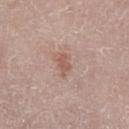<record>
  <biopsy_status>not biopsied; imaged during a skin examination</biopsy_status>
  <lesion_size>
    <long_diameter_mm_approx>2.5</long_diameter_mm_approx>
  </lesion_size>
  <image>
    <source>total-body photography crop</source>
    <field_of_view_mm>15</field_of_view_mm>
  </image>
  <automated_metrics>
    <shape_asymmetry>0.4</shape_asymmetry>
    <cielab_L>55</cielab_L>
    <cielab_a>20</cielab_a>
    <cielab_b>25</cielab_b>
    <vs_skin_darker_L>9.0</vs_skin_darker_L>
    <vs_skin_contrast_norm>6.0</vs_skin_contrast_norm>
    <color_variation_0_10>0.5</color_variation_0_10>
    <peripheral_color_asymmetry>0.5</peripheral_color_asymmetry>
  </automated_metrics>
  <lighting>white-light</lighting>
  <patient>
    <sex>female</sex>
    <age_approx>65</age_approx>
  </patient>
  <site>left lower leg</site>
</record>